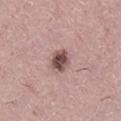Q: Is there a histopathology result?
A: total-body-photography surveillance lesion; no biopsy
Q: Patient demographics?
A: female, aged approximately 40
Q: How large is the lesion?
A: ~3 mm (longest diameter)
Q: What kind of image is this?
A: ~15 mm tile from a whole-body skin photo
Q: What lighting was used for the tile?
A: white-light
Q: Automated lesion metrics?
A: a nevus-likeness score of about 70/100 and a lesion-detection confidence of about 100/100
Q: What is the anatomic site?
A: the right lower leg